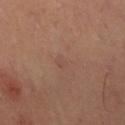Clinical impression:
Recorded during total-body skin imaging; not selected for excision or biopsy.
Clinical summary:
An algorithmic analysis of the crop reported a footprint of about 2 mm², a shape eccentricity near 0.65, and a symmetry-axis asymmetry near 0.3. It also reported a mean CIELAB color near L≈46 a*≈19 b*≈25 and a lesion-to-skin contrast of about 3.5 (normalized; higher = more distinct). A close-up tile cropped from a whole-body skin photograph, about 15 mm across. Captured under cross-polarized illumination. The lesion is located on the right thigh. A female patient, aged 38–42. Longest diameter approximately 1.5 mm.